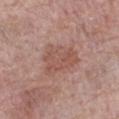<tbp_lesion>
<biopsy_status>not biopsied; imaged during a skin examination</biopsy_status>
</tbp_lesion>